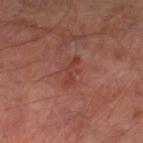Assessment:
The lesion was photographed on a routine skin check and not biopsied; there is no pathology result.
Clinical summary:
The patient is a male approximately 65 years of age. The lesion is on the right lower leg. This is a cross-polarized tile. Approximately 3.5 mm at its widest. This image is a 15 mm lesion crop taken from a total-body photograph. An algorithmic analysis of the crop reported a lesion area of about 4 mm², an outline eccentricity of about 0.9 (0 = round, 1 = elongated), and a symmetry-axis asymmetry near 0.5. And it measured internal color variation of about 0 on a 0–10 scale and a peripheral color-asymmetry measure near 0. The analysis additionally found a classifier nevus-likeness of about 0/100 and a detector confidence of about 100 out of 100 that the crop contains a lesion.